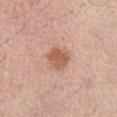Q: Is there a histopathology result?
A: catalogued during a skin exam; not biopsied
Q: Who is the patient?
A: male, aged approximately 70
Q: How was the tile lit?
A: white-light illumination
Q: How was this image acquired?
A: total-body-photography crop, ~15 mm field of view
Q: What is the anatomic site?
A: the abdomen